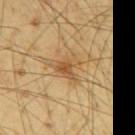| feature | finding |
|---|---|
| follow-up | catalogued during a skin exam; not biopsied |
| imaging modality | ~15 mm tile from a whole-body skin photo |
| illumination | cross-polarized illumination |
| TBP lesion metrics | an area of roughly 7.5 mm² and an eccentricity of roughly 0.5; a classifier nevus-likeness of about 65/100 and a detector confidence of about 100 out of 100 that the crop contains a lesion |
| site | the mid back |
| subject | male, aged 63–67 |
| lesion diameter | about 4 mm |